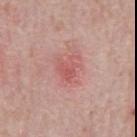{"biopsy_status": "not biopsied; imaged during a skin examination"}